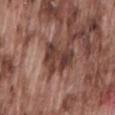Impression:
No biopsy was performed on this lesion — it was imaged during a full skin examination and was not determined to be concerning.
Clinical summary:
Approximately 4.5 mm at its widest. A male subject, approximately 75 years of age. The lesion is located on the lower back. A 15 mm close-up extracted from a 3D total-body photography capture. Imaged with white-light lighting.